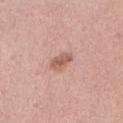The lesion is located on the abdomen.
A female subject approximately 50 years of age.
A region of skin cropped from a whole-body photographic capture, roughly 15 mm wide.